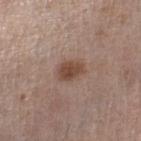| field | value |
|---|---|
| follow-up | imaged on a skin check; not biopsied |
| anatomic site | the right thigh |
| lesion diameter | ~3 mm (longest diameter) |
| image source | ~15 mm crop, total-body skin-cancer survey |
| tile lighting | white-light |
| subject | female, aged 58 to 62 |